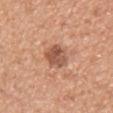notes=total-body-photography surveillance lesion; no biopsy | image source=total-body-photography crop, ~15 mm field of view | patient=female, aged around 40 | body site=the chest.